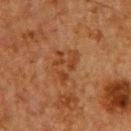{"biopsy_status": "not biopsied; imaged during a skin examination", "site": "upper back", "image": {"source": "total-body photography crop", "field_of_view_mm": 15}, "automated_metrics": {"border_irregularity_0_10": 6.0, "color_variation_0_10": 4.0, "peripheral_color_asymmetry": 1.5, "lesion_detection_confidence_0_100": 100}, "lighting": "cross-polarized", "patient": {"sex": "male", "age_approx": 60}, "lesion_size": {"long_diameter_mm_approx": 4.0}}